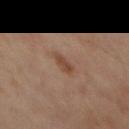| feature | finding |
|---|---|
| site | the mid back |
| patient | male, in their mid- to late 50s |
| diameter | ≈2.5 mm |
| image | ~15 mm crop, total-body skin-cancer survey |
| lighting | cross-polarized |